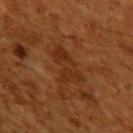Assessment: Imaged during a routine full-body skin examination; the lesion was not biopsied and no histopathology is available.